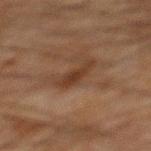Assessment:
The lesion was photographed on a routine skin check and not biopsied; there is no pathology result.
Acquisition and patient details:
The lesion-visualizer software estimated an average lesion color of about L≈28 a*≈16 b*≈25 (CIELAB), roughly 7 lightness units darker than nearby skin, and a lesion-to-skin contrast of about 8 (normalized; higher = more distinct). The analysis additionally found an automated nevus-likeness rating near 10 out of 100. The lesion is on the mid back. A 15 mm close-up extracted from a 3D total-body photography capture. About 3.5 mm across. Captured under cross-polarized illumination. The patient is a male roughly 65 years of age.